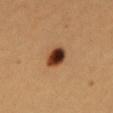Background:
The lesion is on the chest. An algorithmic analysis of the crop reported an area of roughly 6.5 mm² and a shape eccentricity near 0.7. And it measured a lesion-to-skin contrast of about 15 (normalized; higher = more distinct). And it measured a within-lesion color-variation index near 10/10 and peripheral color asymmetry of about 3. And it measured a classifier nevus-likeness of about 100/100 and lesion-presence confidence of about 100/100. A close-up tile cropped from a whole-body skin photograph, about 15 mm across. The tile uses cross-polarized illumination. A female subject aged 28–32.